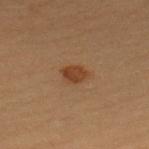Q: Was this lesion biopsied?
A: total-body-photography surveillance lesion; no biopsy
Q: What lighting was used for the tile?
A: cross-polarized
Q: What kind of image is this?
A: ~15 mm tile from a whole-body skin photo
Q: Where on the body is the lesion?
A: the upper back
Q: How large is the lesion?
A: ~2.5 mm (longest diameter)
Q: What are the patient's age and sex?
A: female, aged 28 to 32
Q: What did automated image analysis measure?
A: a mean CIELAB color near L≈32 a*≈18 b*≈28 and a lesion–skin lightness drop of about 8; border irregularity of about 2 on a 0–10 scale, a within-lesion color-variation index near 2/10, and peripheral color asymmetry of about 1; a lesion-detection confidence of about 100/100